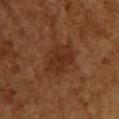body site: the chest | illumination: cross-polarized | image: total-body-photography crop, ~15 mm field of view | lesion diameter: about 4 mm | TBP lesion metrics: a footprint of about 11 mm², an eccentricity of roughly 0.65, and a shape-asymmetry score of about 0.15 (0 = symmetric); an automated nevus-likeness rating near 15 out of 100 and a lesion-detection confidence of about 100/100 | subject: female, in their mid- to late 50s.